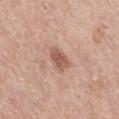workup=no biopsy performed (imaged during a skin exam) | lesion diameter=~3 mm (longest diameter) | imaging modality=15 mm crop, total-body photography | patient=female, aged 63 to 67 | illumination=white-light | body site=the right thigh.